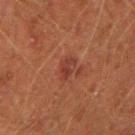The lesion was tiled from a total-body skin photograph and was not biopsied. This image is a 15 mm lesion crop taken from a total-body photograph. A male subject aged 43–47. Located on the right forearm. Imaged with cross-polarized lighting. About 3 mm across.